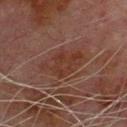{"biopsy_status": "not biopsied; imaged during a skin examination", "patient": {"sex": "male", "age_approx": 80}, "image": {"source": "total-body photography crop", "field_of_view_mm": 15}, "lighting": "cross-polarized", "automated_metrics": {"area_mm2_approx": 13.0, "eccentricity": 0.85, "shape_asymmetry": 0.25}, "lesion_size": {"long_diameter_mm_approx": 5.5}, "site": "chest"}